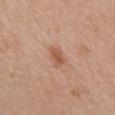No biopsy was performed on this lesion — it was imaged during a full skin examination and was not determined to be concerning.
Located on the chest.
An algorithmic analysis of the crop reported a shape eccentricity near 0.8. And it measured a lesion color around L≈56 a*≈22 b*≈32 in CIELAB and about 9 CIELAB-L* units darker than the surrounding skin.
The subject is a female approximately 50 years of age.
A roughly 15 mm field-of-view crop from a total-body skin photograph.
Longest diameter approximately 3 mm.